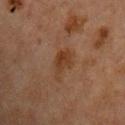Background: A 15 mm close-up tile from a total-body photography series done for melanoma screening. The lesion is on the upper back. The subject is a male in their mid- to late 60s. Measured at roughly 3.5 mm in maximum diameter. This is a cross-polarized tile.